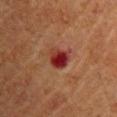This lesion was catalogued during total-body skin photography and was not selected for biopsy. A female patient, aged approximately 70. A 15 mm close-up extracted from a 3D total-body photography capture. Automated tile analysis of the lesion measured an area of roughly 6.5 mm², an outline eccentricity of about 0.35 (0 = round, 1 = elongated), and a symmetry-axis asymmetry near 0.2. It also reported a classifier nevus-likeness of about 0/100 and a lesion-detection confidence of about 100/100. The lesion's longest dimension is about 3 mm. The lesion is on the right upper arm. Captured under cross-polarized illumination.